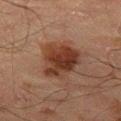The lesion was photographed on a routine skin check and not biopsied; there is no pathology result. From the right thigh. A male patient, aged approximately 65. The recorded lesion diameter is about 5 mm. This image is a 15 mm lesion crop taken from a total-body photograph.